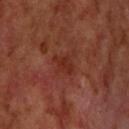{"biopsy_status": "not biopsied; imaged during a skin examination", "patient": {"sex": "male", "age_approx": 70}, "automated_metrics": {"border_irregularity_0_10": 3.0, "color_variation_0_10": 2.0, "peripheral_color_asymmetry": 0.5, "nevus_likeness_0_100": 0, "lesion_detection_confidence_0_100": 100}, "lesion_size": {"long_diameter_mm_approx": 3.0}, "site": "head or neck", "image": {"source": "total-body photography crop", "field_of_view_mm": 15}, "lighting": "cross-polarized"}